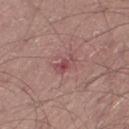  biopsy_status: not biopsied; imaged during a skin examination